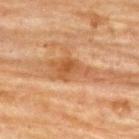This lesion was catalogued during total-body skin photography and was not selected for biopsy. Automated image analysis of the tile measured a shape eccentricity near 0.9 and a symmetry-axis asymmetry near 0.4. The tile uses cross-polarized illumination. A 15 mm crop from a total-body photograph taken for skin-cancer surveillance. On the back. The subject is a female approximately 80 years of age.